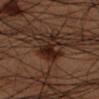biopsy status — imaged on a skin check; not biopsied
diameter — ≈5.5 mm
anatomic site — the left lower leg
imaging modality — 15 mm crop, total-body photography
automated lesion analysis — about 8 CIELAB-L* units darker than the surrounding skin and a normalized border contrast of about 11; radial color variation of about 0.5
subject — male, about 50 years old